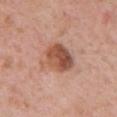biopsy status = total-body-photography surveillance lesion; no biopsy
size = ~4.5 mm (longest diameter)
patient = female, in their 40s
imaging modality = ~15 mm crop, total-body skin-cancer survey
tile lighting = white-light
body site = the chest